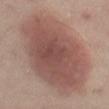Recorded during total-body skin imaging; not selected for excision or biopsy.
This image is a 15 mm lesion crop taken from a total-body photograph.
The lesion is on the left thigh.
The patient is a female aged approximately 55.
Automated tile analysis of the lesion measured a shape eccentricity near 0.8 and a shape-asymmetry score of about 0.1 (0 = symmetric). It also reported an automated nevus-likeness rating near 100 out of 100 and a detector confidence of about 100 out of 100 that the crop contains a lesion.
Approximately 12 mm at its widest.
The tile uses cross-polarized illumination.